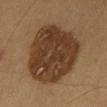Context:
The recorded lesion diameter is about 9.5 mm. A male subject aged 58 to 62. On the leg. Captured under cross-polarized illumination. A close-up tile cropped from a whole-body skin photograph, about 15 mm across.